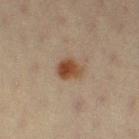workup = imaged on a skin check; not biopsied | lighting = cross-polarized illumination | diameter = about 3 mm | anatomic site = the left thigh | imaging modality = total-body-photography crop, ~15 mm field of view | subject = female, about 20 years old.